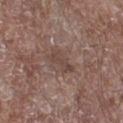<tbp_lesion>
<biopsy_status>not biopsied; imaged during a skin examination</biopsy_status>
<site>right lower leg</site>
<automated_metrics>
  <nevus_likeness_0_100>0</nevus_likeness_0_100>
  <lesion_detection_confidence_0_100>100</lesion_detection_confidence_0_100>
</automated_metrics>
<lesion_size>
  <long_diameter_mm_approx>3.5</long_diameter_mm_approx>
</lesion_size>
<patient>
  <sex>male</sex>
  <age_approx>70</age_approx>
</patient>
<image>
  <source>total-body photography crop</source>
  <field_of_view_mm>15</field_of_view_mm>
</image>
</tbp_lesion>